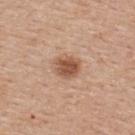Captured under white-light illumination.
A male patient approximately 55 years of age.
Located on the back.
This image is a 15 mm lesion crop taken from a total-body photograph.
Approximately 3 mm at its widest.